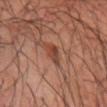{
  "biopsy_status": "not biopsied; imaged during a skin examination",
  "image": {
    "source": "total-body photography crop",
    "field_of_view_mm": 15
  },
  "site": "left forearm",
  "patient": {
    "sex": "male",
    "age_approx": 65
  }
}